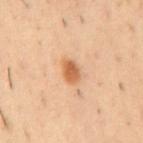Findings:
- workup · imaged on a skin check; not biopsied
- location · the mid back
- illumination · cross-polarized
- size · ≈3 mm
- subject · male, aged approximately 55
- image source · 15 mm crop, total-body photography